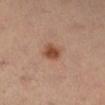Recorded during total-body skin imaging; not selected for excision or biopsy.
A lesion tile, about 15 mm wide, cut from a 3D total-body photograph.
The tile uses cross-polarized illumination.
The lesion is located on the right lower leg.
A female patient, aged 33–37.